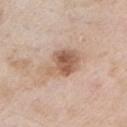Clinical impression: Imaged during a routine full-body skin examination; the lesion was not biopsied and no histopathology is available. Acquisition and patient details: The lesion is on the left upper arm. Cropped from a whole-body photographic skin survey; the tile spans about 15 mm. A male patient, about 55 years old.